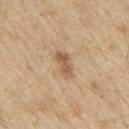Clinical impression:
Imaged during a routine full-body skin examination; the lesion was not biopsied and no histopathology is available.
Context:
The tile uses white-light illumination. A male subject aged 68 to 72. Longest diameter approximately 3.5 mm. The lesion is on the arm. A region of skin cropped from a whole-body photographic capture, roughly 15 mm wide. The lesion-visualizer software estimated a mean CIELAB color near L≈57 a*≈17 b*≈33 and a lesion-to-skin contrast of about 7 (normalized; higher = more distinct).